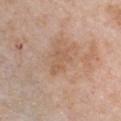This lesion was catalogued during total-body skin photography and was not selected for biopsy. The lesion is located on the chest. A female patient, aged 43–47. Imaged with white-light lighting. Measured at roughly 3.5 mm in maximum diameter. Cropped from a whole-body photographic skin survey; the tile spans about 15 mm. The total-body-photography lesion software estimated a color-variation rating of about 1/10.